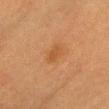Q: Was this lesion biopsied?
A: catalogued during a skin exam; not biopsied
Q: What kind of image is this?
A: total-body-photography crop, ~15 mm field of view
Q: What are the patient's age and sex?
A: female, aged 53 to 57
Q: How large is the lesion?
A: ~2.5 mm (longest diameter)
Q: What did automated image analysis measure?
A: a footprint of about 3.5 mm² and a shape eccentricity near 0.8; a mean CIELAB color near L≈45 a*≈21 b*≈36, roughly 6 lightness units darker than nearby skin, and a lesion-to-skin contrast of about 5.5 (normalized; higher = more distinct); an automated nevus-likeness rating near 5 out of 100
Q: Where on the body is the lesion?
A: the head or neck